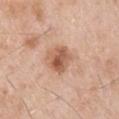Clinical impression: The lesion was photographed on a routine skin check and not biopsied; there is no pathology result. Image and clinical context: On the left upper arm. The subject is a male approximately 55 years of age. Cropped from a whole-body photographic skin survey; the tile spans about 15 mm.